Cropped from a total-body skin-imaging series; the visible field is about 15 mm. A male subject aged approximately 65. The lesion is located on the front of the torso. Captured under cross-polarized illumination. The recorded lesion diameter is about 7 mm.
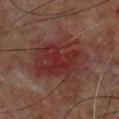Diagnosis:
The lesion was biopsied, and histopathology showed a nodular basal cell carcinoma.